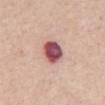Clinical impression: The lesion was tiled from a total-body skin photograph and was not biopsied. Acquisition and patient details: Cropped from a whole-body photographic skin survey; the tile spans about 15 mm. Approximately 3.5 mm at its widest. A female patient in their mid- to late 60s. Imaged with white-light lighting. The lesion is on the abdomen.